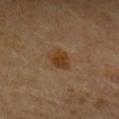{"biopsy_status": "not biopsied; imaged during a skin examination", "image": {"source": "total-body photography crop", "field_of_view_mm": 15}, "patient": {"sex": "male", "age_approx": 65}, "lesion_size": {"long_diameter_mm_approx": 3.0}, "site": "chest"}